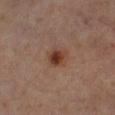{"patient": {"sex": "male", "age_approx": 60}, "image": {"source": "total-body photography crop", "field_of_view_mm": 15}, "site": "left lower leg"}